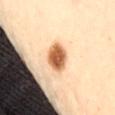{"biopsy_status": "not biopsied; imaged during a skin examination", "lighting": "cross-polarized", "lesion_size": {"long_diameter_mm_approx": 3.5}, "site": "right thigh", "automated_metrics": {"color_variation_0_10": 5.5, "peripheral_color_asymmetry": 1.5}, "image": {"source": "total-body photography crop", "field_of_view_mm": 15}, "patient": {"sex": "male", "age_approx": 65}}